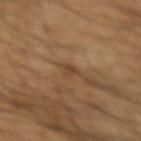<record>
<biopsy_status>not biopsied; imaged during a skin examination</biopsy_status>
<patient>
  <sex>male</sex>
  <age_approx>65</age_approx>
</patient>
<image>
  <source>total-body photography crop</source>
  <field_of_view_mm>15</field_of_view_mm>
</image>
<site>right forearm</site>
</record>